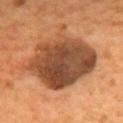Q: Was a biopsy performed?
A: no biopsy performed (imaged during a skin exam)
Q: What did automated image analysis measure?
A: a footprint of about 42 mm², an outline eccentricity of about 0.6 (0 = round, 1 = elongated), and a symmetry-axis asymmetry near 0.2; a mean CIELAB color near L≈43 a*≈21 b*≈32, a lesion–skin lightness drop of about 15, and a normalized border contrast of about 11.5; a border-irregularity rating of about 3/10, a color-variation rating of about 7/10, and radial color variation of about 2; a detector confidence of about 100 out of 100 that the crop contains a lesion
Q: What is the anatomic site?
A: the mid back
Q: Lesion size?
A: ~8 mm (longest diameter)
Q: Who is the patient?
A: male, aged approximately 55
Q: What kind of image is this?
A: 15 mm crop, total-body photography
Q: What lighting was used for the tile?
A: cross-polarized